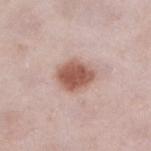Image and clinical context:
A 15 mm crop from a total-body photograph taken for skin-cancer surveillance. On the left lower leg. The total-body-photography lesion software estimated an outline eccentricity of about 0.6 (0 = round, 1 = elongated) and two-axis asymmetry of about 0.1. It also reported a mean CIELAB color near L≈56 a*≈22 b*≈26 and roughly 15 lightness units darker than nearby skin. Approximately 4 mm at its widest. A female subject roughly 30 years of age.